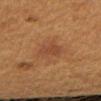biopsy status = catalogued during a skin exam; not biopsied | anatomic site = the right upper arm | subject = female, aged 38 to 42 | imaging modality = 15 mm crop, total-body photography.